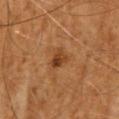No biopsy was performed on this lesion — it was imaged during a full skin examination and was not determined to be concerning. The tile uses cross-polarized illumination. A 15 mm close-up extracted from a 3D total-body photography capture. Longest diameter approximately 2.5 mm. The patient is a male aged around 65.